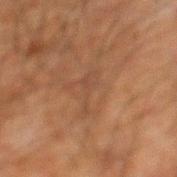Clinical impression:
Captured during whole-body skin photography for melanoma surveillance; the lesion was not biopsied.
Image and clinical context:
Longest diameter approximately 4 mm. From the mid back. The subject is a male aged 58–62. Cropped from a total-body skin-imaging series; the visible field is about 15 mm.